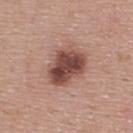{"biopsy_status": "not biopsied; imaged during a skin examination", "site": "back", "image": {"source": "total-body photography crop", "field_of_view_mm": 15}, "lesion_size": {"long_diameter_mm_approx": 5.0}, "lighting": "white-light", "automated_metrics": {"cielab_L": 46, "cielab_a": 23, "cielab_b": 24, "vs_skin_darker_L": 16.0, "vs_skin_contrast_norm": 11.0, "border_irregularity_0_10": 2.0, "color_variation_0_10": 5.5, "nevus_likeness_0_100": 50}, "patient": {"sex": "male", "age_approx": 55}}